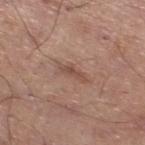No biopsy was performed on this lesion — it was imaged during a full skin examination and was not determined to be concerning. A male subject, aged 68 to 72. The lesion is on the left lower leg. The tile uses white-light illumination. Longest diameter approximately 3.5 mm. A 15 mm crop from a total-body photograph taken for skin-cancer surveillance. An algorithmic analysis of the crop reported an average lesion color of about L≈50 a*≈20 b*≈27 (CIELAB) and a lesion-to-skin contrast of about 6 (normalized; higher = more distinct). The analysis additionally found a classifier nevus-likeness of about 0/100.